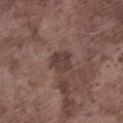Clinical impression: This lesion was catalogued during total-body skin photography and was not selected for biopsy. Context: Imaged with white-light lighting. The lesion is on the left lower leg. A male patient, in their mid- to late 70s. Longest diameter approximately 2.5 mm. The total-body-photography lesion software estimated an eccentricity of roughly 0.25. The analysis additionally found a mean CIELAB color near L≈38 a*≈17 b*≈18, about 8 CIELAB-L* units darker than the surrounding skin, and a normalized border contrast of about 7.5. The analysis additionally found border irregularity of about 2 on a 0–10 scale, internal color variation of about 2.5 on a 0–10 scale, and radial color variation of about 1. A roughly 15 mm field-of-view crop from a total-body skin photograph.